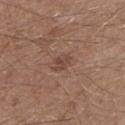Assessment:
Part of a total-body skin-imaging series; this lesion was reviewed on a skin check and was not flagged for biopsy.
Acquisition and patient details:
From the left lower leg. A male patient aged 63–67. Cropped from a whole-body photographic skin survey; the tile spans about 15 mm. Measured at roughly 3 mm in maximum diameter. The tile uses white-light illumination.